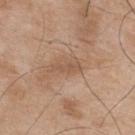Clinical impression: The lesion was tiled from a total-body skin photograph and was not biopsied. Acquisition and patient details: Captured under white-light illumination. A region of skin cropped from a whole-body photographic capture, roughly 15 mm wide. Approximately 3.5 mm at its widest. The lesion is on the mid back. A male subject approximately 75 years of age. The lesion-visualizer software estimated an average lesion color of about L≈54 a*≈18 b*≈31 (CIELAB), about 7 CIELAB-L* units darker than the surrounding skin, and a lesion-to-skin contrast of about 5.5 (normalized; higher = more distinct). The software also gave a nevus-likeness score of about 0/100.